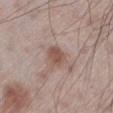  image:
    source: total-body photography crop
    field_of_view_mm: 15
  lesion_size:
    long_diameter_mm_approx: 3.0
  lighting: white-light
  patient:
    sex: male
    age_approx: 60
  site: left lower leg